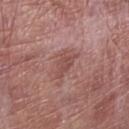Clinical impression: The lesion was tiled from a total-body skin photograph and was not biopsied. Background: A 15 mm crop from a total-body photograph taken for skin-cancer surveillance. The lesion's longest dimension is about 4 mm. A male subject roughly 70 years of age. Located on the leg. This is a white-light tile. An algorithmic analysis of the crop reported a footprint of about 5.5 mm², a shape eccentricity near 0.9, and a shape-asymmetry score of about 0.3 (0 = symmetric). It also reported a border-irregularity index near 3.5/10, a within-lesion color-variation index near 2/10, and radial color variation of about 0.5.